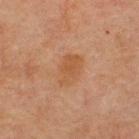Impression:
Imaged during a routine full-body skin examination; the lesion was not biopsied and no histopathology is available.
Image and clinical context:
Located on the upper back. A male subject, aged around 60. Automated tile analysis of the lesion measured a lesion color around L≈44 a*≈19 b*≈31 in CIELAB and roughly 5 lightness units darker than nearby skin. It also reported a classifier nevus-likeness of about 5/100 and a lesion-detection confidence of about 100/100. A 15 mm close-up extracted from a 3D total-body photography capture. The tile uses cross-polarized illumination. Longest diameter approximately 4 mm.